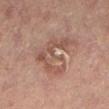Case summary:
– follow-up · imaged on a skin check; not biopsied
– diameter · ~6.5 mm (longest diameter)
– automated metrics · a lesion area of about 19 mm², a shape eccentricity near 0.6, and a shape-asymmetry score of about 0.55 (0 = symmetric); a classifier nevus-likeness of about 0/100 and a lesion-detection confidence of about 100/100
– subject · female, aged around 70
– lighting · cross-polarized illumination
– site · the left lower leg
– acquisition · total-body-photography crop, ~15 mm field of view